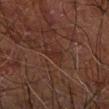{"biopsy_status": "not biopsied; imaged during a skin examination", "site": "arm", "patient": {"sex": "male", "age_approx": 70}, "lighting": "cross-polarized", "image": {"source": "total-body photography crop", "field_of_view_mm": 15}, "lesion_size": {"long_diameter_mm_approx": 2.5}}